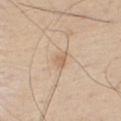Imaged during a routine full-body skin examination; the lesion was not biopsied and no histopathology is available.
Imaged with white-light lighting.
Measured at roughly 2.5 mm in maximum diameter.
The lesion is located on the chest.
This image is a 15 mm lesion crop taken from a total-body photograph.
The patient is a male about 30 years old.
An algorithmic analysis of the crop reported a lesion area of about 3 mm², a shape eccentricity near 0.75, and a symmetry-axis asymmetry near 0.3. And it measured a classifier nevus-likeness of about 0/100 and lesion-presence confidence of about 100/100.